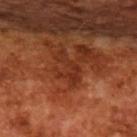Notes:
* biopsy status: total-body-photography surveillance lesion; no biopsy
* patient: male, about 65 years old
* imaging modality: 15 mm crop, total-body photography